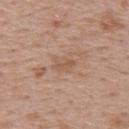Notes:
– workup — catalogued during a skin exam; not biopsied
– automated lesion analysis — an area of roughly 4 mm², a shape eccentricity near 0.8, and a symmetry-axis asymmetry near 0.4; a mean CIELAB color near L≈56 a*≈19 b*≈30, about 7 CIELAB-L* units darker than the surrounding skin, and a normalized lesion–skin contrast near 5; a classifier nevus-likeness of about 0/100 and lesion-presence confidence of about 100/100
– body site — the upper back
– patient — male, in their 70s
– image source — ~15 mm tile from a whole-body skin photo